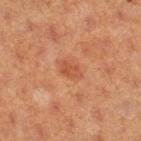notes — imaged on a skin check; not biopsied | diameter — ≈2.5 mm | body site — the left thigh | illumination — cross-polarized | imaging modality — total-body-photography crop, ~15 mm field of view | subject — female, about 40 years old | automated metrics — two-axis asymmetry of about 0.25; a border-irregularity rating of about 2.5/10, a within-lesion color-variation index near 2.5/10, and a peripheral color-asymmetry measure near 1.